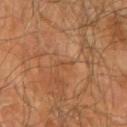The lesion was tiled from a total-body skin photograph and was not biopsied. This image is a 15 mm lesion crop taken from a total-body photograph. The patient is a male aged 63–67. Captured under cross-polarized illumination. The recorded lesion diameter is about 2.5 mm. The lesion is on the left upper arm. Automated tile analysis of the lesion measured a lesion area of about 1.5 mm² and an outline eccentricity of about 0.95 (0 = round, 1 = elongated). The analysis additionally found a classifier nevus-likeness of about 0/100.